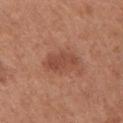About 4 mm across.
A female subject in their mid- to late 60s.
A 15 mm close-up extracted from a 3D total-body photography capture.
The lesion is on the chest.
This is a white-light tile.
Automated image analysis of the tile measured a classifier nevus-likeness of about 30/100 and lesion-presence confidence of about 100/100.